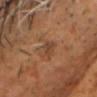Q: Is there a histopathology result?
A: no biopsy performed (imaged during a skin exam)
Q: Patient demographics?
A: male, roughly 50 years of age
Q: Where on the body is the lesion?
A: the head or neck
Q: What is the lesion's diameter?
A: ≈2.5 mm
Q: Illumination type?
A: cross-polarized illumination
Q: What kind of image is this?
A: total-body-photography crop, ~15 mm field of view